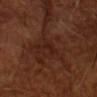Q: Was this lesion biopsied?
A: catalogued during a skin exam; not biopsied
Q: Lesion size?
A: ~2.5 mm (longest diameter)
Q: What lighting was used for the tile?
A: cross-polarized
Q: What are the patient's age and sex?
A: male, in their mid-60s
Q: What kind of image is this?
A: ~15 mm crop, total-body skin-cancer survey